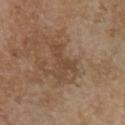The lesion was photographed on a routine skin check and not biopsied; there is no pathology result. A 15 mm close-up extracted from a 3D total-body photography capture. The lesion is located on the front of the torso. The lesion's longest dimension is about 5 mm. A female patient about 65 years old.